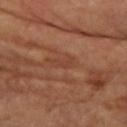size = ≈3 mm; patient = female, in their 60s; acquisition = total-body-photography crop, ~15 mm field of view; body site = the left arm; illumination = cross-polarized illumination.